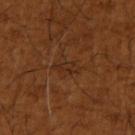subject: male, approximately 65 years of age | size: about 3.5 mm | tile lighting: cross-polarized illumination | image source: ~15 mm tile from a whole-body skin photo | site: the right upper arm.